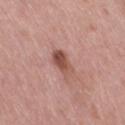workup = no biopsy performed (imaged during a skin exam); subject = female, aged 53–57; site = the leg; lighting = white-light illumination; lesion diameter = about 3.5 mm; image source = 15 mm crop, total-body photography.